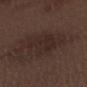Imaged during a routine full-body skin examination; the lesion was not biopsied and no histopathology is available. A lesion tile, about 15 mm wide, cut from a 3D total-body photograph. The lesion is located on the right forearm. Imaged with white-light lighting. A male subject, about 70 years old.